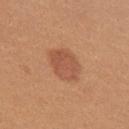Recorded during total-body skin imaging; not selected for excision or biopsy. Approximately 4.5 mm at its widest. A lesion tile, about 15 mm wide, cut from a 3D total-body photograph. A female patient approximately 25 years of age. This is a white-light tile. The lesion is located on the right upper arm.